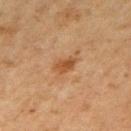Q: Was this lesion biopsied?
A: imaged on a skin check; not biopsied
Q: What is the lesion's diameter?
A: about 3 mm
Q: Lesion location?
A: the right upper arm
Q: Who is the patient?
A: female, aged 43 to 47
Q: What is the imaging modality?
A: ~15 mm tile from a whole-body skin photo
Q: Automated lesion metrics?
A: an average lesion color of about L≈44 a*≈21 b*≈34 (CIELAB) and a normalized border contrast of about 7.5; a border-irregularity index near 3/10, internal color variation of about 3 on a 0–10 scale, and a peripheral color-asymmetry measure near 1
Q: How was the tile lit?
A: cross-polarized illumination